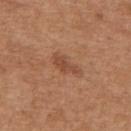This lesion was catalogued during total-body skin photography and was not selected for biopsy.
Located on the upper back.
The lesion-visualizer software estimated a footprint of about 4 mm². The analysis additionally found a mean CIELAB color near L≈47 a*≈23 b*≈32 and roughly 8 lightness units darker than nearby skin. It also reported a lesion-detection confidence of about 100/100.
The recorded lesion diameter is about 3.5 mm.
The tile uses white-light illumination.
A close-up tile cropped from a whole-body skin photograph, about 15 mm across.
The patient is a female approximately 40 years of age.